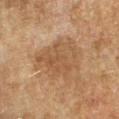The lesion is located on the left forearm. A female subject aged 58–62. The recorded lesion diameter is about 6 mm. The lesion-visualizer software estimated a footprint of about 20 mm². And it measured a border-irregularity rating of about 3.5/10 and a within-lesion color-variation index near 3/10. Imaged with cross-polarized lighting. A 15 mm crop from a total-body photograph taken for skin-cancer surveillance.